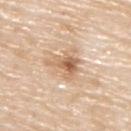Impression:
Imaged during a routine full-body skin examination; the lesion was not biopsied and no histopathology is available.
Background:
A region of skin cropped from a whole-body photographic capture, roughly 15 mm wide. Imaged with white-light lighting. From the upper back. A male patient, roughly 80 years of age.